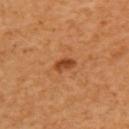Findings:
- workup — catalogued during a skin exam; not biopsied
- imaging modality — total-body-photography crop, ~15 mm field of view
- lighting — cross-polarized
- lesion diameter — ~3 mm (longest diameter)
- subject — female, in their 40s
- location — the left upper arm